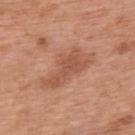| field | value |
|---|---|
| notes | no biopsy performed (imaged during a skin exam) |
| location | the upper back |
| automated metrics | a mean CIELAB color near L≈54 a*≈24 b*≈32, roughly 8 lightness units darker than nearby skin, and a normalized lesion–skin contrast near 6; a nevus-likeness score of about 15/100 and lesion-presence confidence of about 100/100 |
| imaging modality | 15 mm crop, total-body photography |
| lesion diameter | about 7 mm |
| patient | male, aged approximately 70 |